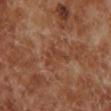Assessment:
Part of a total-body skin-imaging series; this lesion was reviewed on a skin check and was not flagged for biopsy.
Context:
A roughly 15 mm field-of-view crop from a total-body skin photograph. The lesion is located on the left lower leg. A male subject aged 68–72.